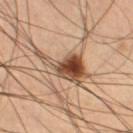• notes — total-body-photography surveillance lesion; no biopsy
• patient — male, aged 63–67
• tile lighting — cross-polarized
• anatomic site — the leg
• automated lesion analysis — a mean CIELAB color near L≈49 a*≈19 b*≈30, a lesion–skin lightness drop of about 16, and a lesion-to-skin contrast of about 11 (normalized; higher = more distinct); a border-irregularity rating of about 6.5/10, a color-variation rating of about 9.5/10, and a peripheral color-asymmetry measure near 3.5; lesion-presence confidence of about 100/100
• image — total-body-photography crop, ~15 mm field of view
• lesion diameter — about 6.5 mm